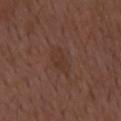{"image": {"source": "total-body photography crop", "field_of_view_mm": 15}, "lighting": "white-light", "patient": {"sex": "male", "age_approx": 50}, "lesion_size": {"long_diameter_mm_approx": 3.5}, "automated_metrics": {"border_irregularity_0_10": 2.5, "nevus_likeness_0_100": 0, "lesion_detection_confidence_0_100": 100}}